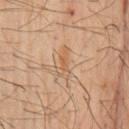follow-up = imaged on a skin check; not biopsied
body site = the chest
patient = male, aged 58–62
lighting = cross-polarized illumination
image = ~15 mm crop, total-body skin-cancer survey
size = about 4 mm